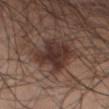  biopsy_status: not biopsied; imaged during a skin examination
  site: chest
  image:
    source: total-body photography crop
    field_of_view_mm: 15
  lesion_size:
    long_diameter_mm_approx: 8.0
  patient:
    sex: male
    age_approx: 55